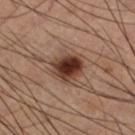Q: Was this lesion biopsied?
A: catalogued during a skin exam; not biopsied
Q: What did automated image analysis measure?
A: a mean CIELAB color near L≈34 a*≈20 b*≈26 and a normalized lesion–skin contrast near 14; a within-lesion color-variation index near 5.5/10 and peripheral color asymmetry of about 1.5
Q: Illumination type?
A: cross-polarized illumination
Q: Who is the patient?
A: male, aged around 65
Q: How was this image acquired?
A: total-body-photography crop, ~15 mm field of view
Q: What is the lesion's diameter?
A: ≈3.5 mm
Q: What is the anatomic site?
A: the left lower leg